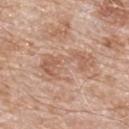On the back. Automated image analysis of the tile measured a shape eccentricity near 0.95 and a symmetry-axis asymmetry near 0.55. The software also gave a lesion color around L≈59 a*≈20 b*≈30 in CIELAB and a normalized border contrast of about 5. The analysis additionally found a border-irregularity index near 8.5/10, internal color variation of about 3 on a 0–10 scale, and a peripheral color-asymmetry measure near 1. About 6.5 mm across. A male subject aged around 80. This is a white-light tile. A region of skin cropped from a whole-body photographic capture, roughly 15 mm wide.